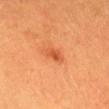Clinical impression:
No biopsy was performed on this lesion — it was imaged during a full skin examination and was not determined to be concerning.
Clinical summary:
Imaged with cross-polarized lighting. A 15 mm crop from a total-body photograph taken for skin-cancer surveillance. From the head or neck. A female subject, roughly 30 years of age. The lesion-visualizer software estimated a lesion area of about 3.5 mm² and a shape eccentricity near 0.8. The software also gave a border-irregularity index near 2/10, a color-variation rating of about 3/10, and peripheral color asymmetry of about 1. It also reported a nevus-likeness score of about 95/100 and lesion-presence confidence of about 100/100. Longest diameter approximately 2.5 mm.